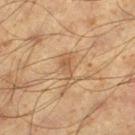| field | value |
|---|---|
| workup | no biopsy performed (imaged during a skin exam) |
| patient | male, aged 58–62 |
| body site | the left thigh |
| imaging modality | ~15 mm crop, total-body skin-cancer survey |